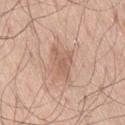{
  "patient": {
    "sex": "male",
    "age_approx": 70
  },
  "lighting": "white-light",
  "site": "right thigh",
  "image": {
    "source": "total-body photography crop",
    "field_of_view_mm": 15
  }
}